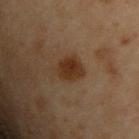Impression:
Recorded during total-body skin imaging; not selected for excision or biopsy.
Background:
A close-up tile cropped from a whole-body skin photograph, about 15 mm across. The lesion is located on the chest. A male patient roughly 55 years of age.